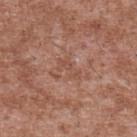The lesion was tiled from a total-body skin photograph and was not biopsied. This image is a 15 mm lesion crop taken from a total-body photograph. A male patient, aged 43 to 47. The lesion is on the upper back.